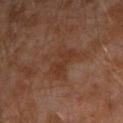Assessment:
Captured during whole-body skin photography for melanoma surveillance; the lesion was not biopsied.
Acquisition and patient details:
The subject is a male aged 28 to 32. On the left arm. Longest diameter approximately 4.5 mm. A region of skin cropped from a whole-body photographic capture, roughly 15 mm wide. This is a cross-polarized tile.